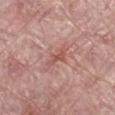Assessment: Captured during whole-body skin photography for melanoma surveillance; the lesion was not biopsied. Background: An algorithmic analysis of the crop reported a footprint of about 3.5 mm² and a symmetry-axis asymmetry near 0.5. The software also gave a border-irregularity index near 5/10, a color-variation rating of about 0/10, and radial color variation of about 0. The software also gave a nevus-likeness score of about 0/100. On the right lower leg. Longest diameter approximately 3 mm. A female subject, approximately 60 years of age. A lesion tile, about 15 mm wide, cut from a 3D total-body photograph.